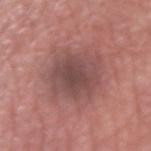The lesion was tiled from a total-body skin photograph and was not biopsied.
A male patient roughly 65 years of age.
Approximately 6 mm at its widest.
On the right upper arm.
An algorithmic analysis of the crop reported a shape eccentricity near 0.6. It also reported an average lesion color of about L≈47 a*≈22 b*≈21 (CIELAB) and a lesion–skin lightness drop of about 9. The software also gave a border-irregularity index near 2.5/10 and radial color variation of about 1. And it measured a nevus-likeness score of about 5/100 and lesion-presence confidence of about 100/100.
A 15 mm crop from a total-body photograph taken for skin-cancer surveillance.